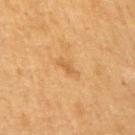Background: Automated image analysis of the tile measured an average lesion color of about L≈54 a*≈20 b*≈40 (CIELAB) and a lesion–skin lightness drop of about 7. The software also gave a border-irregularity index near 3.5/10 and a peripheral color-asymmetry measure near 0. And it measured an automated nevus-likeness rating near 0 out of 100. The lesion is located on the chest. A lesion tile, about 15 mm wide, cut from a 3D total-body photograph. This is a cross-polarized tile. The patient is a male aged approximately 55.